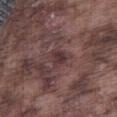The lesion was tiled from a total-body skin photograph and was not biopsied.